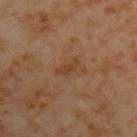Impression: Captured during whole-body skin photography for melanoma surveillance; the lesion was not biopsied. Clinical summary: Automated image analysis of the tile measured a lesion area of about 3 mm², an outline eccentricity of about 0.95 (0 = round, 1 = elongated), and a symmetry-axis asymmetry near 0.4. And it measured internal color variation of about 0 on a 0–10 scale and peripheral color asymmetry of about 0. The lesion's longest dimension is about 3 mm. A 15 mm close-up extracted from a 3D total-body photography capture. The tile uses cross-polarized illumination. A male patient, aged around 60. On the left upper arm.